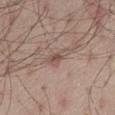notes: no biopsy performed (imaged during a skin exam) | subject: male, aged 53 to 57 | image: 15 mm crop, total-body photography | site: the left thigh.